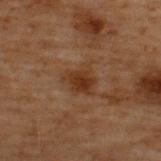A male subject aged approximately 70. A 15 mm close-up extracted from a 3D total-body photography capture. On the upper back. Imaged with cross-polarized lighting. Longest diameter approximately 3.5 mm.